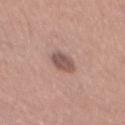| feature | finding |
|---|---|
| biopsy status | no biopsy performed (imaged during a skin exam) |
| lesion size | ~3.5 mm (longest diameter) |
| image source | total-body-photography crop, ~15 mm field of view |
| lighting | white-light |
| site | the back |
| patient | male, aged 53 to 57 |